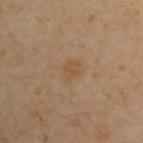Imaged during a routine full-body skin examination; the lesion was not biopsied and no histopathology is available. This image is a 15 mm lesion crop taken from a total-body photograph. Captured under cross-polarized illumination. Located on the left upper arm. A male subject, aged around 40. The total-body-photography lesion software estimated an area of roughly 5.5 mm², a shape eccentricity near 0.7, and a shape-asymmetry score of about 0.3 (0 = symmetric). The analysis additionally found a mean CIELAB color near L≈50 a*≈15 b*≈32 and roughly 5 lightness units darker than nearby skin. The software also gave internal color variation of about 1.5 on a 0–10 scale and peripheral color asymmetry of about 0.5. The lesion's longest dimension is about 3 mm.